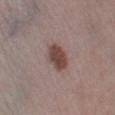Captured during whole-body skin photography for melanoma surveillance; the lesion was not biopsied. The subject is a male aged approximately 60. This image is a 15 mm lesion crop taken from a total-body photograph. Located on the left lower leg. Longest diameter approximately 3.5 mm. The lesion-visualizer software estimated an area of roughly 7.5 mm², a shape eccentricity near 0.8, and a shape-asymmetry score of about 0.15 (0 = symmetric).